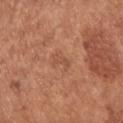– workup · no biopsy performed (imaged during a skin exam)
– subject · male, approximately 55 years of age
– imaging modality · 15 mm crop, total-body photography
– tile lighting · white-light
– lesion diameter · ~3 mm (longest diameter)
– automated lesion analysis · a mean CIELAB color near L≈51 a*≈24 b*≈32, a lesion–skin lightness drop of about 6, and a lesion-to-skin contrast of about 4.5 (normalized; higher = more distinct); border irregularity of about 5 on a 0–10 scale and radial color variation of about 0; a classifier nevus-likeness of about 0/100 and lesion-presence confidence of about 100/100
– site · the front of the torso